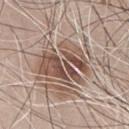Clinical impression: Captured during whole-body skin photography for melanoma surveillance; the lesion was not biopsied. Acquisition and patient details: Automated image analysis of the tile measured a footprint of about 20 mm², an eccentricity of roughly 0.7, and a symmetry-axis asymmetry near 0.35. And it measured an automated nevus-likeness rating near 5 out of 100 and a detector confidence of about 45 out of 100 that the crop contains a lesion. Captured under white-light illumination. On the chest. A male subject, aged around 65. This image is a 15 mm lesion crop taken from a total-body photograph. Approximately 6.5 mm at its widest.